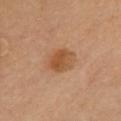{"biopsy_status": "not biopsied; imaged during a skin examination", "patient": {"sex": "male", "age_approx": 55}, "lesion_size": {"long_diameter_mm_approx": 4.0}, "automated_metrics": {"nevus_likeness_0_100": 90}, "image": {"source": "total-body photography crop", "field_of_view_mm": 15}, "lighting": "cross-polarized", "site": "chest"}